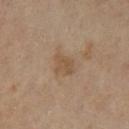| key | value |
|---|---|
| notes | no biopsy performed (imaged during a skin exam) |
| lesion size | about 2.5 mm |
| patient | female, in their 70s |
| body site | the left lower leg |
| lighting | cross-polarized |
| imaging modality | total-body-photography crop, ~15 mm field of view |